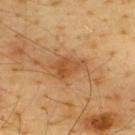No biopsy was performed on this lesion — it was imaged during a full skin examination and was not determined to be concerning.
Automated image analysis of the tile measured an average lesion color of about L≈53 a*≈23 b*≈41 (CIELAB), a lesion–skin lightness drop of about 10, and a normalized lesion–skin contrast near 7. And it measured border irregularity of about 3.5 on a 0–10 scale, a color-variation rating of about 3.5/10, and peripheral color asymmetry of about 1.5. The software also gave a nevus-likeness score of about 65/100 and a detector confidence of about 100 out of 100 that the crop contains a lesion.
A lesion tile, about 15 mm wide, cut from a 3D total-body photograph.
A male patient, roughly 65 years of age.
The lesion is located on the upper back.